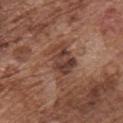Findings:
• workup · imaged on a skin check; not biopsied
• image · total-body-photography crop, ~15 mm field of view
• location · the front of the torso
• subject · male, aged 73 to 77